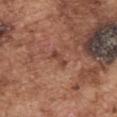biopsy_status: not biopsied; imaged during a skin examination
patient:
  sex: male
  age_approx: 75
automated_metrics:
  area_mm2_approx: 2.5
  eccentricity: 0.9
  cielab_L: 44
  cielab_a: 23
  cielab_b: 28
  vs_skin_darker_L: 8.0
  vs_skin_contrast_norm: 6.5
image:
  source: total-body photography crop
  field_of_view_mm: 15
lesion_size:
  long_diameter_mm_approx: 2.5
site: left upper arm
lighting: white-light